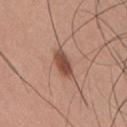Part of a total-body skin-imaging series; this lesion was reviewed on a skin check and was not flagged for biopsy.
This image is a 15 mm lesion crop taken from a total-body photograph.
This is a white-light tile.
From the back.
The recorded lesion diameter is about 3.5 mm.
A male patient, approximately 35 years of age.